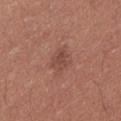Impression:
Captured during whole-body skin photography for melanoma surveillance; the lesion was not biopsied.
Clinical summary:
This is a white-light tile. From the back. A lesion tile, about 15 mm wide, cut from a 3D total-body photograph. A female subject aged 23 to 27.